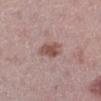Case summary:
- notes — catalogued during a skin exam; not biopsied
- patient — female, roughly 45 years of age
- acquisition — 15 mm crop, total-body photography
- TBP lesion metrics — a lesion area of about 5.5 mm², an eccentricity of roughly 0.8, and a shape-asymmetry score of about 0.2 (0 = symmetric); a mean CIELAB color near L≈51 a*≈20 b*≈23 and about 11 CIELAB-L* units darker than the surrounding skin; border irregularity of about 2.5 on a 0–10 scale, a within-lesion color-variation index near 2.5/10, and radial color variation of about 0.5
- site — the left lower leg
- lesion diameter — ~3.5 mm (longest diameter)